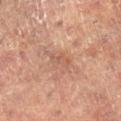biopsy_status: not biopsied; imaged during a skin examination
lighting: cross-polarized
site: leg
lesion_size:
  long_diameter_mm_approx: 3.0
patient:
  sex: female
  age_approx: 80
image:
  source: total-body photography crop
  field_of_view_mm: 15